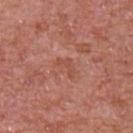Q: Was this lesion biopsied?
A: no biopsy performed (imaged during a skin exam)
Q: How large is the lesion?
A: about 3 mm
Q: How was this image acquired?
A: ~15 mm tile from a whole-body skin photo
Q: What lighting was used for the tile?
A: white-light
Q: Who is the patient?
A: male, aged 63 to 67
Q: What is the anatomic site?
A: the upper back
Q: Automated lesion metrics?
A: an area of roughly 4 mm² and an outline eccentricity of about 0.85 (0 = round, 1 = elongated); a mean CIELAB color near L≈51 a*≈26 b*≈30, a lesion–skin lightness drop of about 5, and a lesion-to-skin contrast of about 4.5 (normalized; higher = more distinct); a border-irregularity rating of about 5.5/10, a within-lesion color-variation index near 0.5/10, and peripheral color asymmetry of about 0